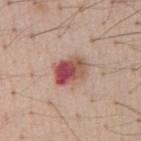The lesion was tiled from a total-body skin photograph and was not biopsied.